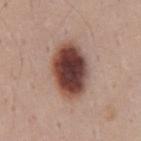Captured during whole-body skin photography for melanoma surveillance; the lesion was not biopsied. A region of skin cropped from a whole-body photographic capture, roughly 15 mm wide. Captured under white-light illumination. The lesion is on the mid back. Automated image analysis of the tile measured a lesion color around L≈42 a*≈22 b*≈23 in CIELAB and a normalized lesion–skin contrast near 15.5. The analysis additionally found a classifier nevus-likeness of about 100/100. About 6.5 mm across. A male subject, approximately 35 years of age.